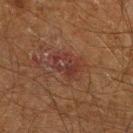Captured during whole-body skin photography for melanoma surveillance; the lesion was not biopsied.
About 3.5 mm across.
Captured under cross-polarized illumination.
A male subject approximately 60 years of age.
This image is a 15 mm lesion crop taken from a total-body photograph.
On the right lower leg.